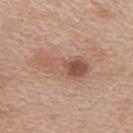follow-up: total-body-photography surveillance lesion; no biopsy | patient: female, in their 40s | size: ≈6 mm | image source: ~15 mm tile from a whole-body skin photo | automated metrics: an automated nevus-likeness rating near 55 out of 100 and a lesion-detection confidence of about 100/100 | illumination: white-light illumination | anatomic site: the right upper arm.